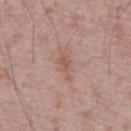Assessment:
Part of a total-body skin-imaging series; this lesion was reviewed on a skin check and was not flagged for biopsy.
Image and clinical context:
Approximately 4 mm at its widest. The tile uses white-light illumination. A male subject roughly 70 years of age. An algorithmic analysis of the crop reported an eccentricity of roughly 0.9 and two-axis asymmetry of about 0.3. And it measured a lesion–skin lightness drop of about 7 and a normalized border contrast of about 6. The analysis additionally found border irregularity of about 4.5 on a 0–10 scale and peripheral color asymmetry of about 0.5. And it measured a nevus-likeness score of about 0/100 and lesion-presence confidence of about 100/100. This image is a 15 mm lesion crop taken from a total-body photograph. On the abdomen.